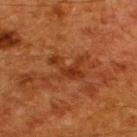follow-up=imaged on a skin check; not biopsied
anatomic site=the upper back
lesion diameter=~4 mm (longest diameter)
image=15 mm crop, total-body photography
patient=male, aged approximately 60
tile lighting=cross-polarized